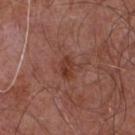A 15 mm crop from a total-body photograph taken for skin-cancer surveillance. A male subject aged 53 to 57. Longest diameter approximately 2.5 mm. Imaged with white-light lighting. Automated image analysis of the tile measured a footprint of about 3.5 mm², an outline eccentricity of about 0.8 (0 = round, 1 = elongated), and two-axis asymmetry of about 0.25. And it measured a mean CIELAB color near L≈37 a*≈25 b*≈28, roughly 8 lightness units darker than nearby skin, and a normalized lesion–skin contrast near 7.5. On the chest.